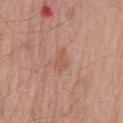notes=no biopsy performed (imaged during a skin exam)
imaging modality=15 mm crop, total-body photography
subject=male, approximately 70 years of age
body site=the mid back